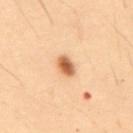anatomic site: the abdomen | image: ~15 mm crop, total-body skin-cancer survey | automated lesion analysis: a border-irregularity rating of about 1/10, internal color variation of about 4 on a 0–10 scale, and peripheral color asymmetry of about 1; a detector confidence of about 100 out of 100 that the crop contains a lesion | tile lighting: cross-polarized illumination | patient: male, aged 53–57.